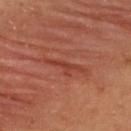Part of a total-body skin-imaging series; this lesion was reviewed on a skin check and was not flagged for biopsy. A female patient, in their mid- to late 30s. Located on the back. The tile uses cross-polarized illumination. A region of skin cropped from a whole-body photographic capture, roughly 15 mm wide.